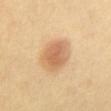notes — no biopsy performed (imaged during a skin exam); location — the abdomen; patient — female, in their mid-60s; acquisition — ~15 mm tile from a whole-body skin photo.